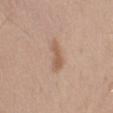Case summary:
• follow-up: catalogued during a skin exam; not biopsied
• diameter: ~4 mm (longest diameter)
• acquisition: 15 mm crop, total-body photography
• lighting: white-light
• subject: male, aged around 45
• site: the right upper arm
• automated metrics: a lesion area of about 5.5 mm², a shape eccentricity near 0.9, and two-axis asymmetry of about 0.35